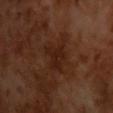Part of a total-body skin-imaging series; this lesion was reviewed on a skin check and was not flagged for biopsy. A male subject roughly 65 years of age. Automated tile analysis of the lesion measured a footprint of about 6 mm² and a symmetry-axis asymmetry near 0.5. The tile uses cross-polarized illumination. A lesion tile, about 15 mm wide, cut from a 3D total-body photograph. Measured at roughly 3.5 mm in maximum diameter.